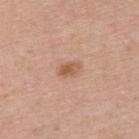Clinical impression: The lesion was tiled from a total-body skin photograph and was not biopsied. Context: A 15 mm close-up tile from a total-body photography series done for melanoma screening. The patient is a female aged 38 to 42. The total-body-photography lesion software estimated an area of roughly 3.5 mm², an outline eccentricity of about 0.8 (0 = round, 1 = elongated), and two-axis asymmetry of about 0.25. And it measured a lesion color around L≈57 a*≈21 b*≈33 in CIELAB and a lesion–skin lightness drop of about 10. And it measured border irregularity of about 2.5 on a 0–10 scale, internal color variation of about 3.5 on a 0–10 scale, and a peripheral color-asymmetry measure near 1. And it measured an automated nevus-likeness rating near 65 out of 100 and a detector confidence of about 100 out of 100 that the crop contains a lesion. The lesion is located on the upper back.